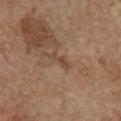workup: no biopsy performed (imaged during a skin exam) | lesion diameter: ≈3 mm | image: ~15 mm tile from a whole-body skin photo | location: the chest | lighting: white-light | patient: female, in their mid-60s.